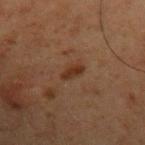Findings:
– follow-up: no biopsy performed (imaged during a skin exam)
– size: ~3 mm (longest diameter)
– location: the left upper arm
– patient: male, aged approximately 60
– image: total-body-photography crop, ~15 mm field of view
– TBP lesion metrics: border irregularity of about 2.5 on a 0–10 scale, a color-variation rating of about 1/10, and a peripheral color-asymmetry measure near 0; a nevus-likeness score of about 70/100 and a detector confidence of about 100 out of 100 that the crop contains a lesion